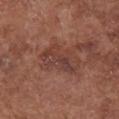<record>
  <biopsy_status>not biopsied; imaged during a skin examination</biopsy_status>
  <image>
    <source>total-body photography crop</source>
    <field_of_view_mm>15</field_of_view_mm>
  </image>
  <lesion_size>
    <long_diameter_mm_approx>5.0</long_diameter_mm_approx>
  </lesion_size>
  <automated_metrics>
    <area_mm2_approx>11.0</area_mm2_approx>
    <eccentricity>0.85</eccentricity>
    <shape_asymmetry>0.3</shape_asymmetry>
    <cielab_L>40</cielab_L>
    <cielab_a>22</cielab_a>
    <cielab_b>24</cielab_b>
    <vs_skin_darker_L>7.0</vs_skin_darker_L>
    <vs_skin_contrast_norm>6.0</vs_skin_contrast_norm>
    <lesion_detection_confidence_0_100>100</lesion_detection_confidence_0_100>
  </automated_metrics>
  <site>chest</site>
  <patient>
    <sex>female</sex>
    <age_approx>75</age_approx>
  </patient>
  <lighting>white-light</lighting>
</record>